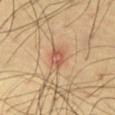The lesion was photographed on a routine skin check and not biopsied; there is no pathology result.
Automated tile analysis of the lesion measured an area of roughly 5 mm², an eccentricity of roughly 0.65, and two-axis asymmetry of about 0.25. And it measured an average lesion color of about L≈58 a*≈24 b*≈33 (CIELAB) and a normalized lesion–skin contrast near 6.5. And it measured radial color variation of about 1.5.
Located on the abdomen.
The patient is a male roughly 65 years of age.
The recorded lesion diameter is about 3 mm.
A 15 mm close-up extracted from a 3D total-body photography capture.